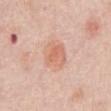<record>
  <biopsy_status>not biopsied; imaged during a skin examination</biopsy_status>
  <lesion_size>
    <long_diameter_mm_approx>4.0</long_diameter_mm_approx>
  </lesion_size>
  <image>
    <source>total-body photography crop</source>
    <field_of_view_mm>15</field_of_view_mm>
  </image>
  <site>front of the torso</site>
  <patient>
    <sex>female</sex>
    <age_approx>65</age_approx>
  </patient>
</record>